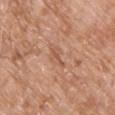No biopsy was performed on this lesion — it was imaged during a full skin examination and was not determined to be concerning. On the chest. A male patient, roughly 80 years of age. A roughly 15 mm field-of-view crop from a total-body skin photograph.